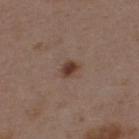  biopsy_status: not biopsied; imaged during a skin examination
  site: back
  image:
    source: total-body photography crop
    field_of_view_mm: 15
  patient:
    sex: female
    age_approx: 45
  lighting: white-light
  automated_metrics:
    vs_skin_darker_L: 11.0
    vs_skin_contrast_norm: 9.0